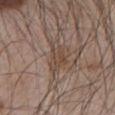Clinical impression: The lesion was tiled from a total-body skin photograph and was not biopsied.